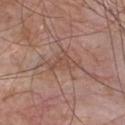follow-up=catalogued during a skin exam; not biopsied | subject=male, roughly 65 years of age | body site=the chest | image=15 mm crop, total-body photography | diameter=~4 mm (longest diameter) | lighting=white-light.